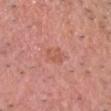size: about 2.5 mm | acquisition: ~15 mm crop, total-body skin-cancer survey | tile lighting: white-light illumination | automated lesion analysis: an outline eccentricity of about 0.7 (0 = round, 1 = elongated) and a shape-asymmetry score of about 0.25 (0 = symmetric); an automated nevus-likeness rating near 0 out of 100 | site: the head or neck | subject: male, about 60 years old.